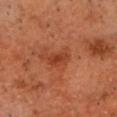Acquisition and patient details:
The patient is a male about 70 years old. From the chest. A lesion tile, about 15 mm wide, cut from a 3D total-body photograph. This is a cross-polarized tile. About 2.5 mm across.